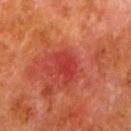Q: Was this lesion biopsied?
A: total-body-photography surveillance lesion; no biopsy
Q: Automated lesion metrics?
A: an area of roughly 6 mm², a shape eccentricity near 0.75, and a shape-asymmetry score of about 0.25 (0 = symmetric); roughly 6 lightness units darker than nearby skin and a normalized lesion–skin contrast near 5.5; a classifier nevus-likeness of about 5/100 and a lesion-detection confidence of about 100/100
Q: Where on the body is the lesion?
A: the left lower leg
Q: Illumination type?
A: cross-polarized illumination
Q: What is the lesion's diameter?
A: ≈3.5 mm
Q: What kind of image is this?
A: ~15 mm tile from a whole-body skin photo
Q: What are the patient's age and sex?
A: male, in their 80s